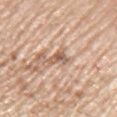Notes:
• notes — no biopsy performed (imaged during a skin exam)
• anatomic site — the left upper arm
• lighting — white-light illumination
• patient — male, aged around 75
• image — 15 mm crop, total-body photography
• lesion size — ~2.5 mm (longest diameter)